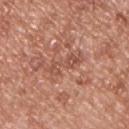follow-up = total-body-photography surveillance lesion; no biopsy
lesion diameter = ~4.5 mm (longest diameter)
anatomic site = the upper back
acquisition = 15 mm crop, total-body photography
patient = male, in their mid-50s